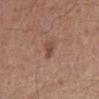A male patient, aged approximately 60. The lesion is on the left lower leg. A lesion tile, about 15 mm wide, cut from a 3D total-body photograph.